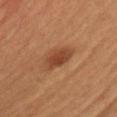biopsy_status: not biopsied; imaged during a skin examination
image:
  source: total-body photography crop
  field_of_view_mm: 15
site: chest
patient:
  sex: male
  age_approx: 50
automated_metrics:
  border_irregularity_0_10: 2.0
  color_variation_0_10: 2.0
  peripheral_color_asymmetry: 0.5
lesion_size:
  long_diameter_mm_approx: 3.5